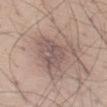- workup · total-body-photography surveillance lesion; no biopsy
- image · ~15 mm tile from a whole-body skin photo
- site · the left thigh
- subject · male, aged approximately 30